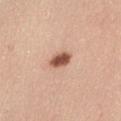workup: total-body-photography surveillance lesion; no biopsy
lesion size: ≈3 mm
site: the abdomen
TBP lesion metrics: a mean CIELAB color near L≈54 a*≈23 b*≈30, roughly 18 lightness units darker than nearby skin, and a lesion-to-skin contrast of about 11.5 (normalized; higher = more distinct); a detector confidence of about 100 out of 100 that the crop contains a lesion
subject: female, roughly 40 years of age
image source: ~15 mm crop, total-body skin-cancer survey